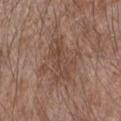<lesion>
  <biopsy_status>not biopsied; imaged during a skin examination</biopsy_status>
  <image>
    <source>total-body photography crop</source>
    <field_of_view_mm>15</field_of_view_mm>
  </image>
  <patient>
    <sex>male</sex>
    <age_approx>55</age_approx>
  </patient>
  <lighting>white-light</lighting>
  <automated_metrics>
    <cielab_L>46</cielab_L>
    <cielab_a>18</cielab_a>
    <cielab_b>26</cielab_b>
    <vs_skin_darker_L>7.0</vs_skin_darker_L>
    <vs_skin_contrast_norm>5.5</vs_skin_contrast_norm>
    <nevus_likeness_0_100>5</nevus_likeness_0_100>
    <lesion_detection_confidence_0_100>100</lesion_detection_confidence_0_100>
  </automated_metrics>
  <site>right forearm</site>
  <lesion_size>
    <long_diameter_mm_approx>6.0</long_diameter_mm_approx>
  </lesion_size>
</lesion>